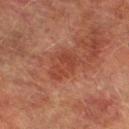The lesion was photographed on a routine skin check and not biopsied; there is no pathology result. Cropped from a whole-body photographic skin survey; the tile spans about 15 mm. A male patient, aged 73 to 77. From the left lower leg. Imaged with cross-polarized lighting. Measured at roughly 4 mm in maximum diameter.